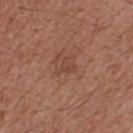notes: no biopsy performed (imaged during a skin exam)
patient: male, aged 53 to 57
site: the upper back
acquisition: ~15 mm tile from a whole-body skin photo